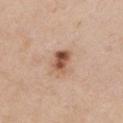automated metrics: an area of roughly 6 mm², an outline eccentricity of about 0.7 (0 = round, 1 = elongated), and a shape-asymmetry score of about 0.2 (0 = symmetric); a border-irregularity rating of about 2/10, a within-lesion color-variation index near 7/10, and radial color variation of about 3; a classifier nevus-likeness of about 90/100 and a detector confidence of about 100 out of 100 that the crop contains a lesion | image: ~15 mm tile from a whole-body skin photo | patient: female, aged 43–47 | tile lighting: white-light | size: ~3 mm (longest diameter) | body site: the chest.